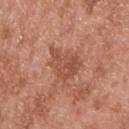No biopsy was performed on this lesion — it was imaged during a full skin examination and was not determined to be concerning. A roughly 15 mm field-of-view crop from a total-body skin photograph. A male patient roughly 55 years of age. Measured at roughly 5 mm in maximum diameter. Located on the upper back. The tile uses white-light illumination.